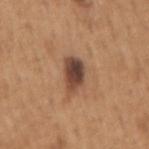follow-up = imaged on a skin check; not biopsied
imaging modality = ~15 mm crop, total-body skin-cancer survey
location = the arm
lighting = white-light
patient = male, in their mid- to late 60s
lesion size = ≈4 mm